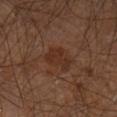follow-up=catalogued during a skin exam; not biopsied
body site=the leg
diameter=about 3 mm
subject=male, about 60 years old
image=~15 mm tile from a whole-body skin photo